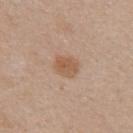Findings:
* follow-up · total-body-photography surveillance lesion; no biopsy
* lighting · white-light illumination
* image · ~15 mm tile from a whole-body skin photo
* lesion size · ≈3 mm
* automated lesion analysis · a lesion area of about 6 mm² and a symmetry-axis asymmetry near 0.2; a border-irregularity index near 2/10, internal color variation of about 2 on a 0–10 scale, and radial color variation of about 1
* body site · the upper back
* subject · female, approximately 40 years of age